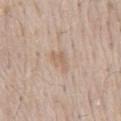Clinical impression: Part of a total-body skin-imaging series; this lesion was reviewed on a skin check and was not flagged for biopsy. Context: Cropped from a whole-body photographic skin survey; the tile spans about 15 mm. A male subject in their mid-60s. The lesion is located on the mid back.